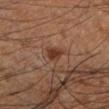Clinical impression: Captured during whole-body skin photography for melanoma surveillance; the lesion was not biopsied.